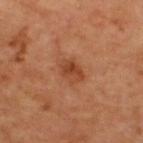{"biopsy_status": "not biopsied; imaged during a skin examination", "site": "upper back", "image": {"source": "total-body photography crop", "field_of_view_mm": 15}, "patient": {"sex": "male", "age_approx": 60}, "lighting": "cross-polarized", "automated_metrics": {"vs_skin_darker_L": 9.0, "vs_skin_contrast_norm": 7.0, "border_irregularity_0_10": 2.0, "color_variation_0_10": 4.0, "peripheral_color_asymmetry": 1.5}, "lesion_size": {"long_diameter_mm_approx": 3.0}}